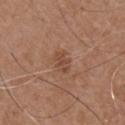Q: Was this lesion biopsied?
A: imaged on a skin check; not biopsied
Q: Patient demographics?
A: male, aged around 75
Q: What is the imaging modality?
A: ~15 mm tile from a whole-body skin photo
Q: What lighting was used for the tile?
A: white-light illumination
Q: Where on the body is the lesion?
A: the chest